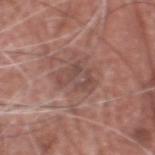Q: Is there a histopathology result?
A: total-body-photography surveillance lesion; no biopsy
Q: Illumination type?
A: white-light illumination
Q: What is the anatomic site?
A: the head or neck
Q: Lesion size?
A: ≈4 mm
Q: Patient demographics?
A: male, aged around 75
Q: How was this image acquired?
A: 15 mm crop, total-body photography
Q: What did automated image analysis measure?
A: border irregularity of about 5.5 on a 0–10 scale, internal color variation of about 4.5 on a 0–10 scale, and peripheral color asymmetry of about 1.5